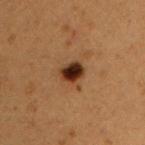Part of a total-body skin-imaging series; this lesion was reviewed on a skin check and was not flagged for biopsy. A 15 mm crop from a total-body photograph taken for skin-cancer surveillance. A male subject aged around 50. An algorithmic analysis of the crop reported a lesion color around L≈25 a*≈18 b*≈25 in CIELAB. The analysis additionally found a classifier nevus-likeness of about 100/100. The tile uses cross-polarized illumination. On the chest. Approximately 3 mm at its widest.